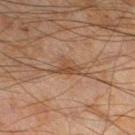  biopsy_status: not biopsied; imaged during a skin examination
  lighting: cross-polarized
  lesion_size:
    long_diameter_mm_approx: 3.0
  automated_metrics:
    border_irregularity_0_10: 5.0
    color_variation_0_10: 2.0
  site: left lower leg
  patient:
    sex: male
    age_approx: 45
  image:
    source: total-body photography crop
    field_of_view_mm: 15